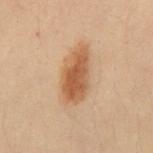| field | value |
|---|---|
| notes | catalogued during a skin exam; not biopsied |
| acquisition | total-body-photography crop, ~15 mm field of view |
| location | the abdomen |
| subject | male, approximately 30 years of age |
| size | ~6 mm (longest diameter) |